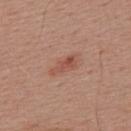follow-up = total-body-photography surveillance lesion; no biopsy | location = the back | imaging modality = total-body-photography crop, ~15 mm field of view | automated lesion analysis = an area of roughly 4 mm² and a shape eccentricity near 0.9; an average lesion color of about L≈51 a*≈24 b*≈29 (CIELAB), roughly 8 lightness units darker than nearby skin, and a normalized lesion–skin contrast near 6.5; border irregularity of about 4 on a 0–10 scale and peripheral color asymmetry of about 0.5; an automated nevus-likeness rating near 35 out of 100 and lesion-presence confidence of about 100/100 | tile lighting = white-light illumination | subject = male, roughly 55 years of age.